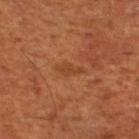Q: How large is the lesion?
A: ~3 mm (longest diameter)
Q: How was this image acquired?
A: ~15 mm tile from a whole-body skin photo
Q: Patient demographics?
A: aged approximately 65
Q: What is the anatomic site?
A: the left lower leg
Q: How was the tile lit?
A: cross-polarized illumination